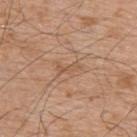Q: Was a biopsy performed?
A: catalogued during a skin exam; not biopsied
Q: What is the anatomic site?
A: the upper back
Q: How was this image acquired?
A: 15 mm crop, total-body photography
Q: Patient demographics?
A: male, about 80 years old
Q: How was the tile lit?
A: white-light
Q: Lesion size?
A: about 2.5 mm